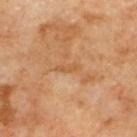Case summary:
– workup: catalogued during a skin exam; not biopsied
– anatomic site: the upper back
– imaging modality: ~15 mm tile from a whole-body skin photo
– lighting: cross-polarized
– subject: male, aged approximately 70
– diameter: about 3 mm
– image-analysis metrics: a border-irregularity rating of about 3.5/10 and a color-variation rating of about 0/10; a nevus-likeness score of about 0/100 and lesion-presence confidence of about 95/100